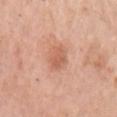The total-body-photography lesion software estimated a footprint of about 4.5 mm². It also reported a normalized lesion–skin contrast near 6. The analysis additionally found an automated nevus-likeness rating near 50 out of 100.
Located on the left upper arm.
The tile uses white-light illumination.
The lesion's longest dimension is about 3 mm.
Cropped from a whole-body photographic skin survey; the tile spans about 15 mm.
The subject is a female approximately 65 years of age.